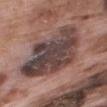Captured during whole-body skin photography for melanoma surveillance; the lesion was not biopsied. Imaged with white-light lighting. Located on the upper back. Cropped from a total-body skin-imaging series; the visible field is about 15 mm. A male patient roughly 70 years of age. Longest diameter approximately 7.5 mm. Automated tile analysis of the lesion measured an average lesion color of about L≈42 a*≈16 b*≈18 (CIELAB), a lesion–skin lightness drop of about 14, and a lesion-to-skin contrast of about 11.5 (normalized; higher = more distinct).